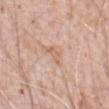Impression:
The lesion was photographed on a routine skin check and not biopsied; there is no pathology result.
Clinical summary:
A lesion tile, about 15 mm wide, cut from a 3D total-body photograph. A male subject, about 80 years old. The lesion is on the abdomen.